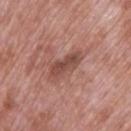Q: Was a biopsy performed?
A: no biopsy performed (imaged during a skin exam)
Q: Where on the body is the lesion?
A: the upper back
Q: What is the imaging modality?
A: 15 mm crop, total-body photography
Q: Who is the patient?
A: male, aged approximately 70
Q: What is the lesion's diameter?
A: ≈4 mm
Q: Automated lesion metrics?
A: a shape eccentricity near 0.85 and a shape-asymmetry score of about 0.2 (0 = symmetric); border irregularity of about 2.5 on a 0–10 scale, a color-variation rating of about 3.5/10, and a peripheral color-asymmetry measure near 1.5; a detector confidence of about 100 out of 100 that the crop contains a lesion